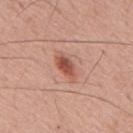Q: Was a biopsy performed?
A: imaged on a skin check; not biopsied
Q: How was this image acquired?
A: total-body-photography crop, ~15 mm field of view
Q: What is the lesion's diameter?
A: ≈3.5 mm
Q: What is the anatomic site?
A: the mid back
Q: What are the patient's age and sex?
A: male, aged 53–57
Q: Automated lesion metrics?
A: a footprint of about 5 mm², an eccentricity of roughly 0.85, and two-axis asymmetry of about 0.25; a border-irregularity rating of about 2.5/10, a color-variation rating of about 4/10, and a peripheral color-asymmetry measure near 1; a nevus-likeness score of about 95/100 and lesion-presence confidence of about 100/100
Q: Illumination type?
A: white-light illumination